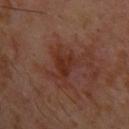Findings:
* workup: total-body-photography surveillance lesion; no biopsy
* patient: male, roughly 30 years of age
* site: the left upper arm
* image source: 15 mm crop, total-body photography